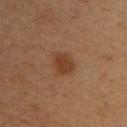No biopsy was performed on this lesion — it was imaged during a full skin examination and was not determined to be concerning. The lesion's longest dimension is about 3 mm. A female subject roughly 40 years of age. This image is a 15 mm lesion crop taken from a total-body photograph. Located on the upper back. This is a cross-polarized tile.